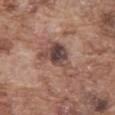The lesion was tiled from a total-body skin photograph and was not biopsied.